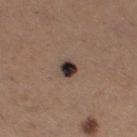Clinical impression: Imaged during a routine full-body skin examination; the lesion was not biopsied and no histopathology is available. Context: A lesion tile, about 15 mm wide, cut from a 3D total-body photograph. Measured at roughly 2.5 mm in maximum diameter. From the right thigh. Automated image analysis of the tile measured a lesion area of about 4 mm², a shape eccentricity near 0.4, and two-axis asymmetry of about 0.2. A female subject aged around 30. The tile uses white-light illumination.